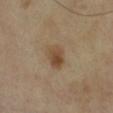Impression: This lesion was catalogued during total-body skin photography and was not selected for biopsy. Context: A region of skin cropped from a whole-body photographic capture, roughly 15 mm wide. Imaged with cross-polarized lighting. A female patient, roughly 70 years of age. Located on the left lower leg.